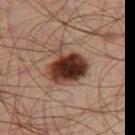{"biopsy_status": "not biopsied; imaged during a skin examination"}